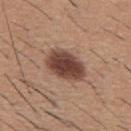notes — catalogued during a skin exam; not biopsied